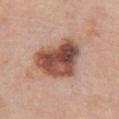biopsy_status: not biopsied; imaged during a skin examination